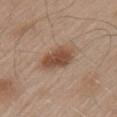Part of a total-body skin-imaging series; this lesion was reviewed on a skin check and was not flagged for biopsy. A male subject in their mid- to late 50s. Longest diameter approximately 4 mm. A 15 mm close-up extracted from a 3D total-body photography capture. From the left upper arm.